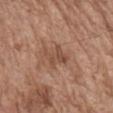Q: Was this lesion biopsied?
A: catalogued during a skin exam; not biopsied
Q: What kind of image is this?
A: ~15 mm tile from a whole-body skin photo
Q: What is the anatomic site?
A: the chest
Q: Patient demographics?
A: male, aged 73 to 77